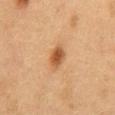Located on the back.
A 15 mm close-up extracted from a 3D total-body photography capture.
A male subject, aged approximately 55.